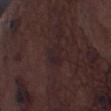This lesion was catalogued during total-body skin photography and was not selected for biopsy. A male patient, about 75 years old. A 15 mm crop from a total-body photograph taken for skin-cancer surveillance. The lesion is located on the left thigh.